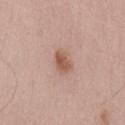workup: imaged on a skin check; not biopsied
anatomic site: the lower back
image source: total-body-photography crop, ~15 mm field of view
subject: male, aged 53–57